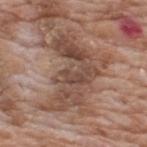The lesion is located on the upper back. Approximately 8.5 mm at its widest. An algorithmic analysis of the crop reported an automated nevus-likeness rating near 0 out of 100 and a detector confidence of about 55 out of 100 that the crop contains a lesion. The tile uses white-light illumination. A male patient approximately 60 years of age. A region of skin cropped from a whole-body photographic capture, roughly 15 mm wide.